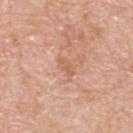| feature | finding |
|---|---|
| follow-up | imaged on a skin check; not biopsied |
| acquisition | 15 mm crop, total-body photography |
| subject | male, approximately 80 years of age |
| TBP lesion metrics | a footprint of about 3 mm², an eccentricity of roughly 0.85, and a symmetry-axis asymmetry near 0.25; an average lesion color of about L≈61 a*≈23 b*≈33 (CIELAB), roughly 7 lightness units darker than nearby skin, and a normalized lesion–skin contrast near 5 |
| lighting | white-light |
| body site | the upper back |
| lesion diameter | ≈2.5 mm |